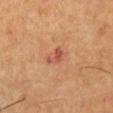Impression: Imaged during a routine full-body skin examination; the lesion was not biopsied and no histopathology is available. Context: A lesion tile, about 15 mm wide, cut from a 3D total-body photograph. The tile uses cross-polarized illumination. A female subject in their 50s. Longest diameter approximately 2.5 mm.